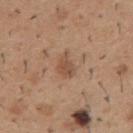<record>
  <patient>
    <sex>male</sex>
    <age_approx>40</age_approx>
  </patient>
  <image>
    <source>total-body photography crop</source>
    <field_of_view_mm>15</field_of_view_mm>
  </image>
  <lighting>white-light</lighting>
  <lesion_size>
    <long_diameter_mm_approx>3.0</long_diameter_mm_approx>
  </lesion_size>
  <site>mid back</site>
</record>